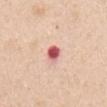Recorded during total-body skin imaging; not selected for excision or biopsy.
A roughly 15 mm field-of-view crop from a total-body skin photograph.
The patient is a male aged around 60.
The lesion is on the mid back.
Captured under white-light illumination.
Automated image analysis of the tile measured an average lesion color of about L≈60 a*≈33 b*≈25 (CIELAB), a lesion–skin lightness drop of about 19, and a lesion-to-skin contrast of about 11 (normalized; higher = more distinct). The analysis additionally found a within-lesion color-variation index near 6.5/10 and peripheral color asymmetry of about 2. The analysis additionally found a classifier nevus-likeness of about 0/100.
Longest diameter approximately 2.5 mm.